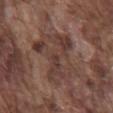Q: Is there a histopathology result?
A: total-body-photography surveillance lesion; no biopsy
Q: How was the tile lit?
A: white-light illumination
Q: Lesion size?
A: about 7 mm
Q: What are the patient's age and sex?
A: male, aged around 75
Q: What is the anatomic site?
A: the front of the torso
Q: What is the imaging modality?
A: total-body-photography crop, ~15 mm field of view